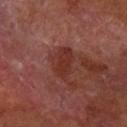No biopsy was performed on this lesion — it was imaged during a full skin examination and was not determined to be concerning. A close-up tile cropped from a whole-body skin photograph, about 15 mm across. The tile uses cross-polarized illumination. A male patient in their 70s. The lesion is located on the right forearm.